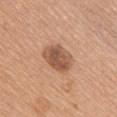Case summary:
– notes: imaged on a skin check; not biopsied
– anatomic site: the chest
– illumination: white-light illumination
– automated metrics: an average lesion color of about L≈54 a*≈22 b*≈32 (CIELAB), a lesion–skin lightness drop of about 13, and a normalized lesion–skin contrast near 8.5
– image source: ~15 mm crop, total-body skin-cancer survey
– patient: female, roughly 60 years of age
– lesion diameter: ~4 mm (longest diameter)